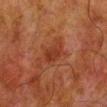  biopsy_status: not biopsied; imaged during a skin examination
  image:
    source: total-body photography crop
    field_of_view_mm: 15
  site: left lower leg
  patient:
    sex: male
    age_approx: 80